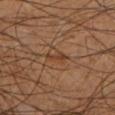Q: Was this lesion biopsied?
A: total-body-photography surveillance lesion; no biopsy
Q: What lighting was used for the tile?
A: cross-polarized
Q: What is the imaging modality?
A: ~15 mm crop, total-body skin-cancer survey
Q: Where on the body is the lesion?
A: the left lower leg
Q: Who is the patient?
A: male, aged around 60